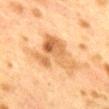No biopsy was performed on this lesion — it was imaged during a full skin examination and was not determined to be concerning.
A female subject, about 40 years old.
Cropped from a total-body skin-imaging series; the visible field is about 15 mm.
Measured at roughly 6.5 mm in maximum diameter.
Located on the back.